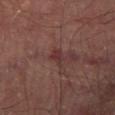Captured during whole-body skin photography for melanoma surveillance; the lesion was not biopsied.
This is a cross-polarized tile.
The recorded lesion diameter is about 3 mm.
An algorithmic analysis of the crop reported a lesion area of about 3 mm², an eccentricity of roughly 0.85, and a shape-asymmetry score of about 0.35 (0 = symmetric). It also reported a mean CIELAB color near L≈32 a*≈20 b*≈18, about 7 CIELAB-L* units darker than the surrounding skin, and a normalized lesion–skin contrast near 7. It also reported a border-irregularity index near 4/10, a color-variation rating of about 0.5/10, and radial color variation of about 0.
The subject is a male aged 68–72.
The lesion is on the leg.
A 15 mm crop from a total-body photograph taken for skin-cancer surveillance.